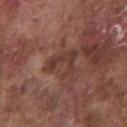Q: Is there a histopathology result?
A: no biopsy performed (imaged during a skin exam)
Q: What is the anatomic site?
A: the chest
Q: What are the patient's age and sex?
A: male, aged approximately 75
Q: What kind of image is this?
A: total-body-photography crop, ~15 mm field of view